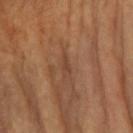Notes:
* notes — no biopsy performed (imaged during a skin exam)
* acquisition — ~15 mm tile from a whole-body skin photo
* body site — the left forearm
* illumination — cross-polarized illumination
* lesion size — ≈2.5 mm
* subject — female, aged around 60